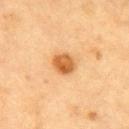site: chest
patient:
  sex: male
  age_approx: 85+
automated_metrics:
  cielab_L: 53
  cielab_a: 23
  cielab_b: 41
  vs_skin_darker_L: 13.0
  vs_skin_contrast_norm: 9.5
  border_irregularity_0_10: 1.0
  peripheral_color_asymmetry: 1.5
image:
  source: total-body photography crop
  field_of_view_mm: 15
lighting: cross-polarized
lesion_size:
  long_diameter_mm_approx: 2.5